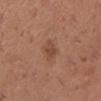A roughly 15 mm field-of-view crop from a total-body skin photograph. The lesion is located on the right lower leg. Imaged with white-light lighting. The patient is a female in their 30s. Measured at roughly 2.5 mm in maximum diameter.